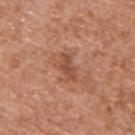notes = no biopsy performed (imaged during a skin exam)